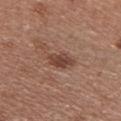Q: Is there a histopathology result?
A: total-body-photography surveillance lesion; no biopsy
Q: What is the lesion's diameter?
A: ≈3.5 mm
Q: How was this image acquired?
A: 15 mm crop, total-body photography
Q: Patient demographics?
A: female, in their 40s
Q: What lighting was used for the tile?
A: white-light
Q: What did automated image analysis measure?
A: a lesion color around L≈42 a*≈21 b*≈26 in CIELAB, roughly 11 lightness units darker than nearby skin, and a normalized border contrast of about 8.5; a border-irregularity index near 3/10, a color-variation rating of about 1.5/10, and a peripheral color-asymmetry measure near 0.5
Q: What is the anatomic site?
A: the chest